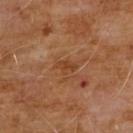Assessment:
Captured during whole-body skin photography for melanoma surveillance; the lesion was not biopsied.
Context:
The lesion is on the chest. The patient is a male in their 60s. The lesion-visualizer software estimated two-axis asymmetry of about 0.25. The software also gave border irregularity of about 3 on a 0–10 scale, a color-variation rating of about 1/10, and peripheral color asymmetry of about 0. It also reported lesion-presence confidence of about 100/100. Cropped from a total-body skin-imaging series; the visible field is about 15 mm. Captured under cross-polarized illumination. Approximately 2.5 mm at its widest.